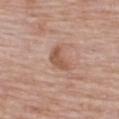Q: Was a biopsy performed?
A: total-body-photography surveillance lesion; no biopsy
Q: Lesion location?
A: the upper back
Q: Automated lesion metrics?
A: a footprint of about 5 mm², a shape eccentricity near 0.8, and a shape-asymmetry score of about 0.4 (0 = symmetric); a border-irregularity index near 4/10, internal color variation of about 1.5 on a 0–10 scale, and radial color variation of about 0.5
Q: What lighting was used for the tile?
A: white-light illumination
Q: What are the patient's age and sex?
A: female, in their 70s
Q: How was this image acquired?
A: 15 mm crop, total-body photography